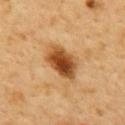<tbp_lesion>
  <biopsy_status>not biopsied; imaged during a skin examination</biopsy_status>
  <lesion_size>
    <long_diameter_mm_approx>5.0</long_diameter_mm_approx>
  </lesion_size>
  <automated_metrics>
    <cielab_L>50</cielab_L>
    <cielab_a>24</cielab_a>
    <cielab_b>42</cielab_b>
    <vs_skin_darker_L>17.0</vs_skin_darker_L>
    <color_variation_0_10>8.5</color_variation_0_10>
  </automated_metrics>
  <lighting>cross-polarized</lighting>
  <image>
    <source>total-body photography crop</source>
    <field_of_view_mm>15</field_of_view_mm>
  </image>
  <site>back</site>
  <patient>
    <sex>male</sex>
    <age_approx>50</age_approx>
  </patient>
</tbp_lesion>